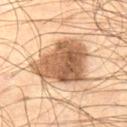The lesion-visualizer software estimated an area of roughly 30 mm², a shape eccentricity near 0.6, and two-axis asymmetry of about 0.3. It also reported a border-irregularity rating of about 3.5/10, internal color variation of about 5.5 on a 0–10 scale, and radial color variation of about 2. Located on the left thigh. A region of skin cropped from a whole-body photographic capture, roughly 15 mm wide. A male subject, roughly 55 years of age. The tile uses cross-polarized illumination.